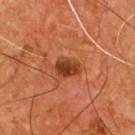Assessment:
Imaged during a routine full-body skin examination; the lesion was not biopsied and no histopathology is available.
Image and clinical context:
The tile uses cross-polarized illumination. A 15 mm close-up tile from a total-body photography series done for melanoma screening. A male patient aged approximately 55. The lesion is located on the front of the torso. Longest diameter approximately 3 mm.